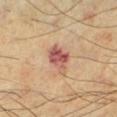workup — imaged on a skin check; not biopsied
automated metrics — a lesion color around L≈52 a*≈24 b*≈26 in CIELAB, a lesion–skin lightness drop of about 12, and a normalized lesion–skin contrast near 9.5; a border-irregularity rating of about 3/10 and a within-lesion color-variation index near 5.5/10; a classifier nevus-likeness of about 10/100 and a detector confidence of about 100 out of 100 that the crop contains a lesion
anatomic site — the left lower leg
lesion diameter — ~4 mm (longest diameter)
image source — 15 mm crop, total-body photography
tile lighting — cross-polarized
patient — male, aged approximately 70